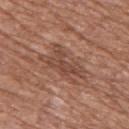No biopsy was performed on this lesion — it was imaged during a full skin examination and was not determined to be concerning. A male subject, aged 58–62. The lesion is located on the front of the torso. Approximately 6 mm at its widest. Captured under white-light illumination. A 15 mm crop from a total-body photograph taken for skin-cancer surveillance.